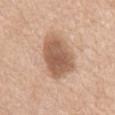The lesion was photographed on a routine skin check and not biopsied; there is no pathology result. A male patient roughly 75 years of age. A region of skin cropped from a whole-body photographic capture, roughly 15 mm wide. From the chest.